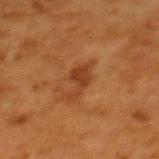  site: upper back
  lighting: cross-polarized
  lesion_size:
    long_diameter_mm_approx: 4.0
  patient:
    sex: female
    age_approx: 50
  image:
    source: total-body photography crop
    field_of_view_mm: 15
  automated_metrics:
    vs_skin_darker_L: 7.0
    vs_skin_contrast_norm: 7.0
    nevus_likeness_0_100: 50
    lesion_detection_confidence_0_100: 100